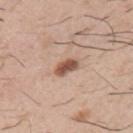The lesion was tiled from a total-body skin photograph and was not biopsied. Cropped from a whole-body photographic skin survey; the tile spans about 15 mm. A male patient in their 50s. Located on the arm. An algorithmic analysis of the crop reported a footprint of about 4.5 mm², an eccentricity of roughly 0.75, and two-axis asymmetry of about 0.25. It also reported roughly 15 lightness units darker than nearby skin and a normalized lesion–skin contrast near 10. And it measured a border-irregularity index near 2.5/10, internal color variation of about 3.5 on a 0–10 scale, and peripheral color asymmetry of about 1. And it measured an automated nevus-likeness rating near 95 out of 100 and a lesion-detection confidence of about 100/100.